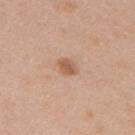The lesion was photographed on a routine skin check and not biopsied; there is no pathology result. Imaged with white-light lighting. From the back. A female subject, roughly 50 years of age. A region of skin cropped from a whole-body photographic capture, roughly 15 mm wide.